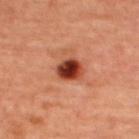- follow-up · total-body-photography surveillance lesion; no biopsy
- patient · female, aged around 45
- location · the back
- image source · total-body-photography crop, ~15 mm field of view